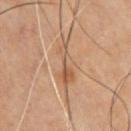{
  "biopsy_status": "not biopsied; imaged during a skin examination",
  "image": {
    "source": "total-body photography crop",
    "field_of_view_mm": 15
  },
  "lighting": "cross-polarized",
  "patient": {
    "sex": "male",
    "age_approx": 55
  },
  "automated_metrics": {
    "border_irregularity_0_10": 5.5,
    "color_variation_0_10": 5.5,
    "nevus_likeness_0_100": 0,
    "lesion_detection_confidence_0_100": 80
  },
  "site": "chest"
}